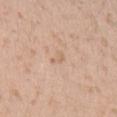Clinical impression:
The lesion was tiled from a total-body skin photograph and was not biopsied.
Image and clinical context:
A 15 mm crop from a total-body photograph taken for skin-cancer surveillance. The lesion's longest dimension is about 2.5 mm. The lesion is on the arm. The subject is a male in their 30s. The tile uses white-light illumination. An algorithmic analysis of the crop reported an area of roughly 2 mm² and two-axis asymmetry of about 0.6. It also reported an automated nevus-likeness rating near 0 out of 100 and a detector confidence of about 100 out of 100 that the crop contains a lesion.